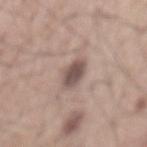Q: Was this lesion biopsied?
A: catalogued during a skin exam; not biopsied
Q: What is the imaging modality?
A: ~15 mm tile from a whole-body skin photo
Q: Patient demographics?
A: male, aged 53–57
Q: Automated lesion metrics?
A: a border-irregularity rating of about 2/10, a color-variation rating of about 2/10, and peripheral color asymmetry of about 0.5
Q: How was the tile lit?
A: white-light
Q: What is the lesion's diameter?
A: ≈3 mm
Q: Lesion location?
A: the mid back